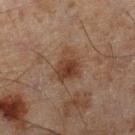Recorded during total-body skin imaging; not selected for excision or biopsy.
From the right lower leg.
A region of skin cropped from a whole-body photographic capture, roughly 15 mm wide.
A male subject, about 45 years old.